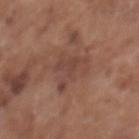Clinical impression: The lesion was tiled from a total-body skin photograph and was not biopsied. Acquisition and patient details: Located on the back. A female patient about 75 years old. Cropped from a total-body skin-imaging series; the visible field is about 15 mm. Longest diameter approximately 5 mm. The tile uses white-light illumination.